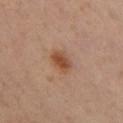Findings:
– biopsy status: catalogued during a skin exam; not biopsied
– lesion diameter: ~3 mm (longest diameter)
– subject: female, about 40 years old
– image source: ~15 mm crop, total-body skin-cancer survey
– anatomic site: the left thigh
– lighting: cross-polarized illumination